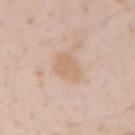notes=imaged on a skin check; not biopsied